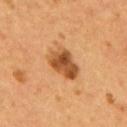| field | value |
|---|---|
| notes | catalogued during a skin exam; not biopsied |
| subject | male, aged approximately 55 |
| image source | total-body-photography crop, ~15 mm field of view |
| automated metrics | an outline eccentricity of about 0.8 (0 = round, 1 = elongated) and a symmetry-axis asymmetry near 0.2 |
| diameter | ~4.5 mm (longest diameter) |
| tile lighting | cross-polarized illumination |
| location | the back |